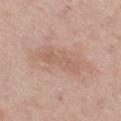The lesion was tiled from a total-body skin photograph and was not biopsied. This is a white-light tile. From the right thigh. A roughly 15 mm field-of-view crop from a total-body skin photograph. A male patient in their mid- to late 70s.